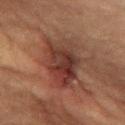biopsy status: no biopsy performed (imaged during a skin exam)
subject: female, in their 70s
automated lesion analysis: lesion-presence confidence of about 95/100
site: the back
image: total-body-photography crop, ~15 mm field of view
lesion size: about 6.5 mm